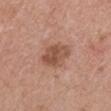Clinical impression: Imaged during a routine full-body skin examination; the lesion was not biopsied and no histopathology is available. Clinical summary: This is a white-light tile. The lesion's longest dimension is about 4 mm. Located on the right upper arm. A 15 mm close-up extracted from a 3D total-body photography capture. Automated tile analysis of the lesion measured a footprint of about 10 mm² and a symmetry-axis asymmetry near 0.2. And it measured a lesion color around L≈52 a*≈22 b*≈30 in CIELAB, roughly 11 lightness units darker than nearby skin, and a lesion-to-skin contrast of about 7.5 (normalized; higher = more distinct). It also reported border irregularity of about 2 on a 0–10 scale, a color-variation rating of about 4/10, and a peripheral color-asymmetry measure near 1.5. A female patient, about 45 years old.